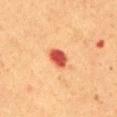Recorded during total-body skin imaging; not selected for excision or biopsy. The tile uses cross-polarized illumination. A 15 mm crop from a total-body photograph taken for skin-cancer surveillance. Approximately 3 mm at its widest. On the abdomen. A female subject in their 50s.